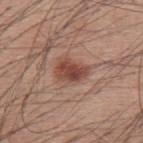Part of a total-body skin-imaging series; this lesion was reviewed on a skin check and was not flagged for biopsy. The lesion is on the upper back. A male subject, in their 60s. This image is a 15 mm lesion crop taken from a total-body photograph.